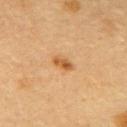| feature | finding |
|---|---|
| automated lesion analysis | a shape eccentricity near 0.85 and a symmetry-axis asymmetry near 0.25; a mean CIELAB color near L≈51 a*≈21 b*≈39, a lesion–skin lightness drop of about 10, and a lesion-to-skin contrast of about 8 (normalized; higher = more distinct) |
| illumination | cross-polarized |
| lesion diameter | ~2.5 mm (longest diameter) |
| body site | the upper back |
| imaging modality | ~15 mm tile from a whole-body skin photo |
| patient | female, aged 58–62 |